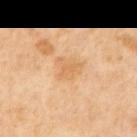{"biopsy_status": "not biopsied; imaged during a skin examination"}